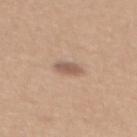Captured during whole-body skin photography for melanoma surveillance; the lesion was not biopsied. Cropped from a total-body skin-imaging series; the visible field is about 15 mm. About 3 mm across. The lesion is on the mid back. The subject is a female about 40 years old.